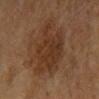A lesion tile, about 15 mm wide, cut from a 3D total-body photograph. The patient is a female in their mid-50s. About 5.5 mm across. Automated tile analysis of the lesion measured a lesion color around L≈28 a*≈16 b*≈26 in CIELAB, about 6 CIELAB-L* units darker than the surrounding skin, and a normalized lesion–skin contrast near 6.5. Captured under cross-polarized illumination. Located on the left forearm.